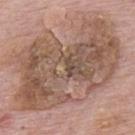acquisition: 15 mm crop, total-body photography; anatomic site: the upper back; patient: male, about 75 years old.